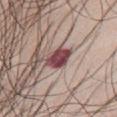follow-up = catalogued during a skin exam; not biopsied | body site = the front of the torso | acquisition = ~15 mm crop, total-body skin-cancer survey | image-analysis metrics = a lesion color around L≈43 a*≈26 b*≈16 in CIELAB, about 19 CIELAB-L* units darker than the surrounding skin, and a normalized border contrast of about 14; border irregularity of about 2.5 on a 0–10 scale, a color-variation rating of about 3.5/10, and a peripheral color-asymmetry measure near 1 | subject = male, aged approximately 65 | illumination = white-light.